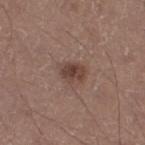• notes: no biopsy performed (imaged during a skin exam)
• image source: total-body-photography crop, ~15 mm field of view
• patient: male, approximately 45 years of age
• anatomic site: the left lower leg
• tile lighting: white-light illumination
• lesion size: ≈3 mm